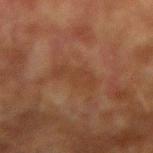The lesion was tiled from a total-body skin photograph and was not biopsied.
A 15 mm close-up extracted from a 3D total-body photography capture.
The patient is a male aged 73–77.
From the arm.